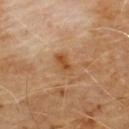The lesion was photographed on a routine skin check and not biopsied; there is no pathology result. A region of skin cropped from a whole-body photographic capture, roughly 15 mm wide. A male subject, aged around 60. The lesion is on the front of the torso.